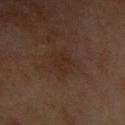The lesion was tiled from a total-body skin photograph and was not biopsied. This image is a 15 mm lesion crop taken from a total-body photograph. Imaged with cross-polarized lighting. Located on the left forearm. An algorithmic analysis of the crop reported a footprint of about 6 mm², an eccentricity of roughly 0.6, and two-axis asymmetry of about 0.25. The analysis additionally found an average lesion color of about L≈23 a*≈14 b*≈21 (CIELAB), a lesion–skin lightness drop of about 4, and a normalized lesion–skin contrast near 5. And it measured border irregularity of about 2.5 on a 0–10 scale, a within-lesion color-variation index near 1.5/10, and a peripheral color-asymmetry measure near 0.5. And it measured a classifier nevus-likeness of about 0/100 and a detector confidence of about 100 out of 100 that the crop contains a lesion. The patient is a female aged 58 to 62.